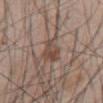notes: catalogued during a skin exam; not biopsied | patient: male, in their mid-40s | image-analysis metrics: an area of roughly 6.5 mm² and an outline eccentricity of about 0.65 (0 = round, 1 = elongated); border irregularity of about 3 on a 0–10 scale, internal color variation of about 4.5 on a 0–10 scale, and a peripheral color-asymmetry measure near 1.5; a nevus-likeness score of about 25/100 | lighting: white-light illumination | size: ~3.5 mm (longest diameter) | image: ~15 mm tile from a whole-body skin photo | location: the abdomen.